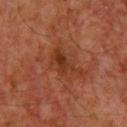Assessment: Part of a total-body skin-imaging series; this lesion was reviewed on a skin check and was not flagged for biopsy. Acquisition and patient details: Approximately 4.5 mm at its widest. The lesion-visualizer software estimated a lesion area of about 6.5 mm² and a shape-asymmetry score of about 0.45 (0 = symmetric). The analysis additionally found an average lesion color of about L≈31 a*≈24 b*≈30 (CIELAB) and a lesion-to-skin contrast of about 7.5 (normalized; higher = more distinct). A 15 mm crop from a total-body photograph taken for skin-cancer surveillance. The lesion is located on the head or neck. A male subject, roughly 60 years of age.